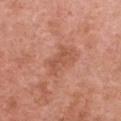Impression:
The lesion was photographed on a routine skin check and not biopsied; there is no pathology result.
Acquisition and patient details:
A female subject, aged around 50. The lesion's longest dimension is about 3.5 mm. The lesion-visualizer software estimated a border-irregularity index near 5/10 and a within-lesion color-variation index near 1.5/10. And it measured a classifier nevus-likeness of about 0/100 and lesion-presence confidence of about 100/100. A 15 mm close-up extracted from a 3D total-body photography capture. From the chest. The tile uses white-light illumination.